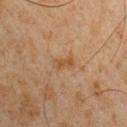This image is a 15 mm lesion crop taken from a total-body photograph. The lesion is on the chest. The subject is a male aged 58 to 62.